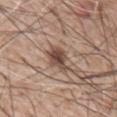Captured during whole-body skin photography for melanoma surveillance; the lesion was not biopsied.
A male subject approximately 60 years of age.
Imaged with white-light lighting.
Cropped from a whole-body photographic skin survey; the tile spans about 15 mm.
From the mid back.